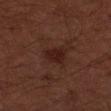Imaged during a routine full-body skin examination; the lesion was not biopsied and no histopathology is available. From the arm. A male subject approximately 60 years of age. A region of skin cropped from a whole-body photographic capture, roughly 15 mm wide.